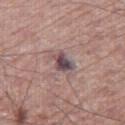Impression:
Part of a total-body skin-imaging series; this lesion was reviewed on a skin check and was not flagged for biopsy.
Clinical summary:
The lesion is located on the right thigh. A male patient about 65 years old. A 15 mm close-up extracted from a 3D total-body photography capture.